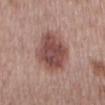Recorded during total-body skin imaging; not selected for excision or biopsy.
The recorded lesion diameter is about 6 mm.
This is a white-light tile.
A roughly 15 mm field-of-view crop from a total-body skin photograph.
The subject is a female in their mid- to late 60s.
On the mid back.
Automated image analysis of the tile measured a lesion area of about 20 mm² and a shape eccentricity near 0.65. The software also gave about 13 CIELAB-L* units darker than the surrounding skin. And it measured border irregularity of about 2 on a 0–10 scale, a within-lesion color-variation index near 4.5/10, and a peripheral color-asymmetry measure near 1.5. It also reported an automated nevus-likeness rating near 60 out of 100 and a lesion-detection confidence of about 100/100.